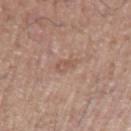The subject is a male approximately 70 years of age.
From the arm.
A 15 mm close-up extracted from a 3D total-body photography capture.